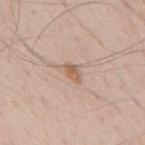Captured during whole-body skin photography for melanoma surveillance; the lesion was not biopsied. A male subject, in their 50s. A lesion tile, about 15 mm wide, cut from a 3D total-body photograph. The total-body-photography lesion software estimated border irregularity of about 3 on a 0–10 scale, a color-variation rating of about 2/10, and radial color variation of about 0.5. The lesion is located on the mid back. Longest diameter approximately 3 mm. Imaged with white-light lighting.